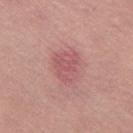follow-up = imaged on a skin check; not biopsied | location = the right thigh | patient = female, aged approximately 65 | lighting = white-light | image = ~15 mm crop, total-body skin-cancer survey | lesion size = ~4 mm (longest diameter).